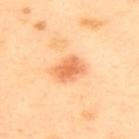notes: total-body-photography surveillance lesion; no biopsy | patient: female, aged approximately 40 | lighting: cross-polarized | size: ≈3.5 mm | imaging modality: ~15 mm crop, total-body skin-cancer survey | body site: the upper back.